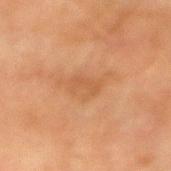| key | value |
|---|---|
| biopsy status | no biopsy performed (imaged during a skin exam) |
| image source | 15 mm crop, total-body photography |
| subject | male, about 75 years old |
| anatomic site | the right upper arm |
| lesion size | ≈3 mm |
| tile lighting | cross-polarized |